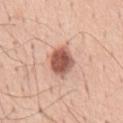{"biopsy_status": "not biopsied; imaged during a skin examination", "site": "mid back", "image": {"source": "total-body photography crop", "field_of_view_mm": 15}, "lesion_size": {"long_diameter_mm_approx": 4.0}, "patient": {"sex": "male", "age_approx": 55}, "lighting": "white-light", "automated_metrics": {"color_variation_0_10": 5.0, "peripheral_color_asymmetry": 1.5, "nevus_likeness_0_100": 95, "lesion_detection_confidence_0_100": 100}}